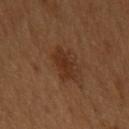Notes:
– notes — no biopsy performed (imaged during a skin exam)